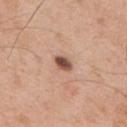The lesion was tiled from a total-body skin photograph and was not biopsied.
Imaged with white-light lighting.
A 15 mm crop from a total-body photograph taken for skin-cancer surveillance.
The subject is a male in their mid- to late 50s.
The lesion is on the upper back.
The recorded lesion diameter is about 2.5 mm.